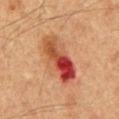{"patient": {"sex": "male", "age_approx": 60}, "lighting": "cross-polarized", "image": {"source": "total-body photography crop", "field_of_view_mm": 15}, "automated_metrics": {"cielab_L": 45, "cielab_a": 30, "cielab_b": 33, "border_irregularity_0_10": 2.5, "color_variation_0_10": 10.0}, "site": "mid back"}